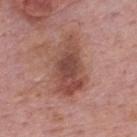This lesion was catalogued during total-body skin photography and was not selected for biopsy.
A 15 mm close-up tile from a total-body photography series done for melanoma screening.
Imaged with white-light lighting.
A male subject, aged around 75.
The lesion is on the upper back.
The total-body-photography lesion software estimated a lesion area of about 20 mm², a shape eccentricity near 0.85, and two-axis asymmetry of about 0.3. The analysis additionally found a lesion color around L≈48 a*≈23 b*≈26 in CIELAB, about 11 CIELAB-L* units darker than the surrounding skin, and a lesion-to-skin contrast of about 8 (normalized; higher = more distinct). The software also gave an automated nevus-likeness rating near 30 out of 100 and a lesion-detection confidence of about 100/100.
Measured at roughly 6.5 mm in maximum diameter.